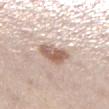The lesion was photographed on a routine skin check and not biopsied; there is no pathology result. The subject is a female aged 28–32. On the right lower leg. A 15 mm crop from a total-body photograph taken for skin-cancer surveillance. The tile uses white-light illumination. About 3.5 mm across.